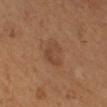Impression:
The lesion was tiled from a total-body skin photograph and was not biopsied.
Context:
The lesion is located on the right lower leg. This image is a 15 mm lesion crop taken from a total-body photograph. The subject is a female aged approximately 55.